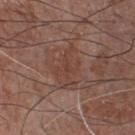  patient:
    sex: male
    age_approx: 75
  automated_metrics:
    eccentricity: 0.8
    shape_asymmetry: 0.5
    vs_skin_contrast_norm: 5.0
    border_irregularity_0_10: 8.5
    peripheral_color_asymmetry: 0.5
  site: chest
  image:
    source: total-body photography crop
    field_of_view_mm: 15
  lighting: white-light
  lesion_size:
    long_diameter_mm_approx: 4.5